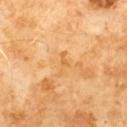Impression: No biopsy was performed on this lesion — it was imaged during a full skin examination and was not determined to be concerning. Context: From the chest. A lesion tile, about 15 mm wide, cut from a 3D total-body photograph. A male patient, roughly 60 years of age. Measured at roughly 3 mm in maximum diameter. Imaged with cross-polarized lighting.